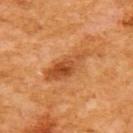Captured during whole-body skin photography for melanoma surveillance; the lesion was not biopsied. Longest diameter approximately 6 mm. Located on the back. Captured under cross-polarized illumination. The patient is a female in their mid- to late 50s. This image is a 15 mm lesion crop taken from a total-body photograph.